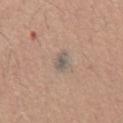The lesion was photographed on a routine skin check and not biopsied; there is no pathology result. The total-body-photography lesion software estimated a border-irregularity index near 3/10 and internal color variation of about 2.5 on a 0–10 scale. The lesion is on the abdomen. A roughly 15 mm field-of-view crop from a total-body skin photograph. The tile uses white-light illumination. Approximately 2.5 mm at its widest. A male subject, roughly 60 years of age.